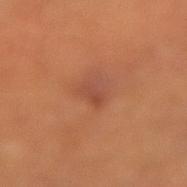Q: Was this lesion biopsied?
A: imaged on a skin check; not biopsied
Q: What is the imaging modality?
A: ~15 mm tile from a whole-body skin photo
Q: Illumination type?
A: cross-polarized illumination
Q: What is the lesion's diameter?
A: ≈2 mm
Q: What is the anatomic site?
A: the left forearm
Q: What did automated image analysis measure?
A: a lesion color around L≈45 a*≈26 b*≈31 in CIELAB, about 6 CIELAB-L* units darker than the surrounding skin, and a normalized lesion–skin contrast near 5.5; a nevus-likeness score of about 0/100 and a detector confidence of about 100 out of 100 that the crop contains a lesion
Q: What are the patient's age and sex?
A: male, about 55 years old